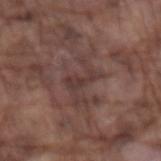<case>
  <biopsy_status>not biopsied; imaged during a skin examination</biopsy_status>
  <lighting>white-light</lighting>
  <patient>
    <sex>male</sex>
    <age_approx>75</age_approx>
  </patient>
  <automated_metrics>
    <area_mm2_approx>4.0</area_mm2_approx>
    <eccentricity>0.9</eccentricity>
    <cielab_L>36</cielab_L>
    <cielab_a>18</cielab_a>
    <cielab_b>19</cielab_b>
    <vs_skin_darker_L>7.0</vs_skin_darker_L>
    <border_irregularity_0_10>5.5</border_irregularity_0_10>
    <nevus_likeness_0_100>0</nevus_likeness_0_100>
    <lesion_detection_confidence_0_100>80</lesion_detection_confidence_0_100>
  </automated_metrics>
  <site>left forearm</site>
  <lesion_size>
    <long_diameter_mm_approx>3.5</long_diameter_mm_approx>
  </lesion_size>
  <image>
    <source>total-body photography crop</source>
    <field_of_view_mm>15</field_of_view_mm>
  </image>
</case>